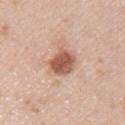biopsy status: imaged on a skin check; not biopsied | patient: male, roughly 40 years of age | image source: ~15 mm crop, total-body skin-cancer survey | anatomic site: the upper back | automated lesion analysis: an area of roughly 8.5 mm², a shape eccentricity near 0.6, and two-axis asymmetry of about 0.2; an average lesion color of about L≈57 a*≈23 b*≈30 (CIELAB), a lesion–skin lightness drop of about 15, and a lesion-to-skin contrast of about 9.5 (normalized; higher = more distinct); a border-irregularity rating of about 2/10, internal color variation of about 3.5 on a 0–10 scale, and peripheral color asymmetry of about 1; a classifier nevus-likeness of about 100/100 and lesion-presence confidence of about 100/100 | illumination: white-light illumination | size: ≈3.5 mm.